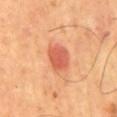{
  "patient": {
    "sex": "male",
    "age_approx": 65
  },
  "lesion_size": {
    "long_diameter_mm_approx": 3.0
  },
  "lighting": "cross-polarized",
  "image": {
    "source": "total-body photography crop",
    "field_of_view_mm": 15
  },
  "automated_metrics": {
    "cielab_L": 60,
    "cielab_a": 34,
    "cielab_b": 38,
    "vs_skin_darker_L": 12.0,
    "vs_skin_contrast_norm": 7.5,
    "nevus_likeness_0_100": 90,
    "lesion_detection_confidence_0_100": 100
  },
  "site": "mid back"
}